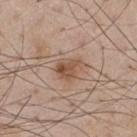follow-up=total-body-photography surveillance lesion; no biopsy
automated metrics=a border-irregularity rating of about 2.5/10, internal color variation of about 4.5 on a 0–10 scale, and radial color variation of about 1.5
size=≈3 mm
acquisition=~15 mm tile from a whole-body skin photo
subject=male, in their mid- to late 50s
anatomic site=the chest